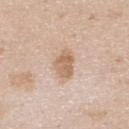Context:
The lesion's longest dimension is about 3.5 mm. Located on the upper back. Cropped from a total-body skin-imaging series; the visible field is about 15 mm. A male patient, aged 23–27. This is a white-light tile.